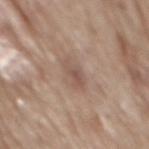biopsy status — catalogued during a skin exam; not biopsied | TBP lesion metrics — an average lesion color of about L≈52 a*≈17 b*≈25 (CIELAB) and a lesion–skin lightness drop of about 8; a border-irregularity rating of about 4/10 and internal color variation of about 2 on a 0–10 scale; lesion-presence confidence of about 100/100 | patient — male, about 70 years old | size — ~3 mm (longest diameter) | acquisition — 15 mm crop, total-body photography | tile lighting — white-light illumination | location — the mid back.